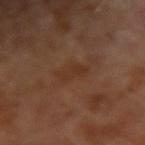biopsy status: imaged on a skin check; not biopsied | imaging modality: total-body-photography crop, ~15 mm field of view | lesion size: ≈3 mm | patient: male, approximately 65 years of age.